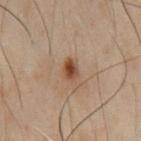No biopsy was performed on this lesion — it was imaged during a full skin examination and was not determined to be concerning.
The subject is a male about 50 years old.
Longest diameter approximately 3 mm.
This image is a 15 mm lesion crop taken from a total-body photograph.
Captured under cross-polarized illumination.
The lesion is on the chest.
Automated tile analysis of the lesion measured roughly 10 lightness units darker than nearby skin. And it measured a border-irregularity rating of about 2.5/10, a color-variation rating of about 3.5/10, and a peripheral color-asymmetry measure near 1.